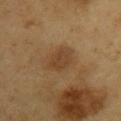| feature | finding |
|---|---|
| biopsy status | imaged on a skin check; not biopsied |
| image | total-body-photography crop, ~15 mm field of view |
| subject | male, aged 58–62 |
| image-analysis metrics | a shape eccentricity near 0.8 and two-axis asymmetry of about 0.2; a lesion color around L≈41 a*≈17 b*≈33 in CIELAB, roughly 8 lightness units darker than nearby skin, and a normalized lesion–skin contrast near 6.5; an automated nevus-likeness rating near 25 out of 100 and lesion-presence confidence of about 100/100 |
| diameter | ≈4 mm |
| illumination | cross-polarized illumination |
| location | the right upper arm |